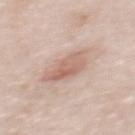<case>
  <biopsy_status>not biopsied; imaged during a skin examination</biopsy_status>
  <patient>
    <sex>female</sex>
    <age_approx>65</age_approx>
  </patient>
  <site>upper back</site>
  <lesion_size>
    <long_diameter_mm_approx>4.5</long_diameter_mm_approx>
  </lesion_size>
  <automated_metrics>
    <nevus_likeness_0_100>55</nevus_likeness_0_100>
    <lesion_detection_confidence_0_100>100</lesion_detection_confidence_0_100>
  </automated_metrics>
  <image>
    <source>total-body photography crop</source>
    <field_of_view_mm>15</field_of_view_mm>
  </image>
</case>